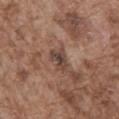Clinical impression: The lesion was tiled from a total-body skin photograph and was not biopsied. Image and clinical context: Measured at roughly 3 mm in maximum diameter. A male patient roughly 75 years of age. The lesion is located on the abdomen. The tile uses white-light illumination. A region of skin cropped from a whole-body photographic capture, roughly 15 mm wide.